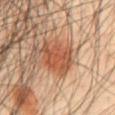Q: Was a biopsy performed?
A: total-body-photography surveillance lesion; no biopsy
Q: Illumination type?
A: cross-polarized illumination
Q: What are the patient's age and sex?
A: male, roughly 45 years of age
Q: How was this image acquired?
A: total-body-photography crop, ~15 mm field of view
Q: What is the lesion's diameter?
A: ~4.5 mm (longest diameter)
Q: What is the anatomic site?
A: the abdomen